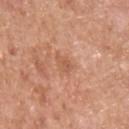<lesion>
  <biopsy_status>not biopsied; imaged during a skin examination</biopsy_status>
  <lighting>white-light</lighting>
  <patient>
    <sex>male</sex>
    <age_approx>60</age_approx>
  </patient>
  <site>back</site>
  <image>
    <source>total-body photography crop</source>
    <field_of_view_mm>15</field_of_view_mm>
  </image>
</lesion>